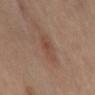The lesion was tiled from a total-body skin photograph and was not biopsied.
The lesion's longest dimension is about 4 mm.
This image is a 15 mm lesion crop taken from a total-body photograph.
This is a cross-polarized tile.
The lesion-visualizer software estimated an outline eccentricity of about 0.85 (0 = round, 1 = elongated) and two-axis asymmetry of about 0.25. The analysis additionally found a mean CIELAB color near L≈47 a*≈20 b*≈28, about 6 CIELAB-L* units darker than the surrounding skin, and a normalized lesion–skin contrast near 5.5. And it measured a classifier nevus-likeness of about 25/100 and a lesion-detection confidence of about 100/100.
Located on the mid back.